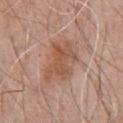Findings:
* patient — male, aged 68 to 72
* TBP lesion metrics — a lesion area of about 15 mm² and an eccentricity of roughly 0.8; an automated nevus-likeness rating near 5 out of 100
* anatomic site — the front of the torso
* image — total-body-photography crop, ~15 mm field of view
* lighting — white-light